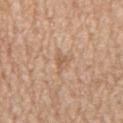This lesion was catalogued during total-body skin photography and was not selected for biopsy. A 15 mm close-up tile from a total-body photography series done for melanoma screening. On the mid back. The subject is a male aged 63–67.